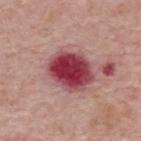| feature | finding |
|---|---|
| notes | imaged on a skin check; not biopsied |
| diameter | ≈5.5 mm |
| tile lighting | white-light |
| subject | male, roughly 65 years of age |
| image source | ~15 mm crop, total-body skin-cancer survey |
| body site | the back |